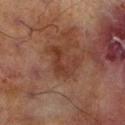Recorded during total-body skin imaging; not selected for excision or biopsy.
An algorithmic analysis of the crop reported an area of roughly 8 mm², a shape eccentricity near 0.7, and a shape-asymmetry score of about 0.5 (0 = symmetric). And it measured a lesion–skin lightness drop of about 6 and a lesion-to-skin contrast of about 6.5 (normalized; higher = more distinct). It also reported border irregularity of about 7.5 on a 0–10 scale and radial color variation of about 0.5. And it measured an automated nevus-likeness rating near 0 out of 100 and a lesion-detection confidence of about 100/100.
From the left lower leg.
Approximately 4 mm at its widest.
A roughly 15 mm field-of-view crop from a total-body skin photograph.
A male patient, aged 68–72.
This is a cross-polarized tile.